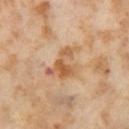Q: How was the tile lit?
A: cross-polarized illumination
Q: How large is the lesion?
A: about 5 mm
Q: Where on the body is the lesion?
A: the left thigh
Q: Patient demographics?
A: female, aged approximately 55
Q: What kind of image is this?
A: ~15 mm crop, total-body skin-cancer survey